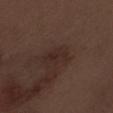Clinical impression:
Part of a total-body skin-imaging series; this lesion was reviewed on a skin check and was not flagged for biopsy.
Context:
The tile uses white-light illumination. The lesion-visualizer software estimated a footprint of about 3.5 mm², an eccentricity of roughly 0.85, and two-axis asymmetry of about 0.45. It also reported a nevus-likeness score of about 0/100. The lesion is located on the right forearm. About 3 mm across. The patient is a male aged approximately 70. A 15 mm close-up extracted from a 3D total-body photography capture.